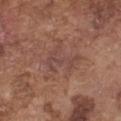workup — no biopsy performed (imaged during a skin exam); anatomic site — the front of the torso; image source — 15 mm crop, total-body photography; subject — male, aged 73 to 77.